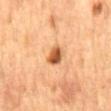Assessment:
Imaged during a routine full-body skin examination; the lesion was not biopsied and no histopathology is available.
Context:
A lesion tile, about 15 mm wide, cut from a 3D total-body photograph. Located on the mid back. The subject is a male about 85 years old or older.